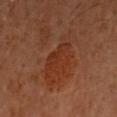The lesion was photographed on a routine skin check and not biopsied; there is no pathology result. Automated tile analysis of the lesion measured a footprint of about 9 mm², an eccentricity of roughly 0.5, and two-axis asymmetry of about 0.35. The software also gave a lesion color around L≈28 a*≈23 b*≈29 in CIELAB. And it measured lesion-presence confidence of about 100/100. This image is a 15 mm lesion crop taken from a total-body photograph. Longest diameter approximately 3.5 mm. From the right arm. This is a cross-polarized tile. A female patient approximately 60 years of age.